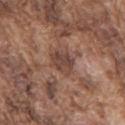The lesion is located on the mid back. This image is a 15 mm lesion crop taken from a total-body photograph. Approximately 3 mm at its widest. The tile uses white-light illumination. A male patient, aged around 75.